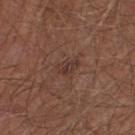| key | value |
|---|---|
| biopsy status | catalogued during a skin exam; not biopsied |
| image-analysis metrics | a footprint of about 4 mm², an eccentricity of roughly 0.8, and two-axis asymmetry of about 0.25; a mean CIELAB color near L≈36 a*≈18 b*≈23, roughly 6 lightness units darker than nearby skin, and a normalized border contrast of about 6; border irregularity of about 2.5 on a 0–10 scale and a peripheral color-asymmetry measure near 1.5; an automated nevus-likeness rating near 0 out of 100 and a lesion-detection confidence of about 100/100 |
| lesion size | ≈3 mm |
| image source | ~15 mm crop, total-body skin-cancer survey |
| anatomic site | the leg |
| illumination | white-light |
| subject | male, aged 63 to 67 |